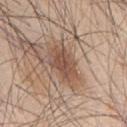Clinical impression: The lesion was photographed on a routine skin check and not biopsied; there is no pathology result. Clinical summary: The lesion is on the mid back. Cropped from a whole-body photographic skin survey; the tile spans about 15 mm. A male subject, in their mid-40s. The recorded lesion diameter is about 4 mm.